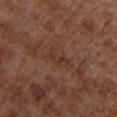Assessment: Imaged during a routine full-body skin examination; the lesion was not biopsied and no histopathology is available. Background: The lesion is on the left lower leg. Captured under cross-polarized illumination. A 15 mm close-up tile from a total-body photography series done for melanoma screening. An algorithmic analysis of the crop reported a lesion area of about 2.5 mm², an eccentricity of roughly 0.9, and two-axis asymmetry of about 0.4. And it measured a border-irregularity rating of about 4.5/10 and a color-variation rating of about 0/10. The recorded lesion diameter is about 3 mm. A male patient, aged 68 to 72.